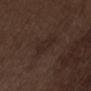<tbp_lesion>
<biopsy_status>not biopsied; imaged during a skin examination</biopsy_status>
<site>abdomen</site>
<patient>
  <sex>male</sex>
  <age_approx>70</age_approx>
</patient>
<lesion_size>
  <long_diameter_mm_approx>3.0</long_diameter_mm_approx>
</lesion_size>
<image>
  <source>total-body photography crop</source>
  <field_of_view_mm>15</field_of_view_mm>
</image>
<lighting>white-light</lighting>
</tbp_lesion>